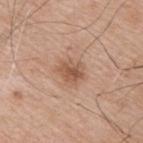biopsy status: no biopsy performed (imaged during a skin exam)
tile lighting: white-light
image-analysis metrics: a lesion area of about 6 mm², an eccentricity of roughly 0.55, and two-axis asymmetry of about 0.25; a mean CIELAB color near L≈55 a*≈21 b*≈31, roughly 10 lightness units darker than nearby skin, and a lesion-to-skin contrast of about 7 (normalized; higher = more distinct); a classifier nevus-likeness of about 35/100 and a lesion-detection confidence of about 100/100
image source: total-body-photography crop, ~15 mm field of view
site: the upper back
patient: male, aged around 75